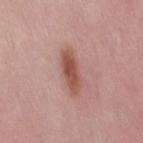| feature | finding |
|---|---|
| workup | imaged on a skin check; not biopsied |
| patient | female, about 50 years old |
| lighting | white-light illumination |
| location | the right thigh |
| image source | ~15 mm crop, total-body skin-cancer survey |